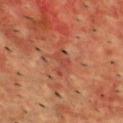Assessment:
No biopsy was performed on this lesion — it was imaged during a full skin examination and was not determined to be concerning.
Acquisition and patient details:
A 15 mm crop from a total-body photograph taken for skin-cancer surveillance. Located on the upper back. A male subject, aged around 55.